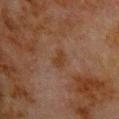| field | value |
|---|---|
| notes | no biopsy performed (imaged during a skin exam) |
| location | the upper back |
| acquisition | total-body-photography crop, ~15 mm field of view |
| subject | male, about 80 years old |